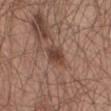follow-up=catalogued during a skin exam; not biopsied
illumination=white-light illumination
site=the abdomen
image source=total-body-photography crop, ~15 mm field of view
lesion size=≈2.5 mm
subject=male, aged 63 to 67